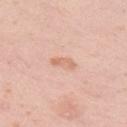The lesion was photographed on a routine skin check and not biopsied; there is no pathology result. This is a white-light tile. A close-up tile cropped from a whole-body skin photograph, about 15 mm across. A female patient aged approximately 30. Located on the right upper arm.